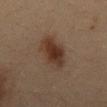Impression: Captured during whole-body skin photography for melanoma surveillance; the lesion was not biopsied.